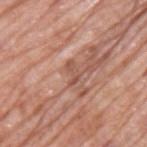Part of a total-body skin-imaging series; this lesion was reviewed on a skin check and was not flagged for biopsy.
A male subject aged 68 to 72.
An algorithmic analysis of the crop reported an area of roughly 3 mm², an outline eccentricity of about 0.85 (0 = round, 1 = elongated), and a shape-asymmetry score of about 0.6 (0 = symmetric). The analysis additionally found a lesion color around L≈53 a*≈23 b*≈28 in CIELAB, about 8 CIELAB-L* units darker than the surrounding skin, and a normalized lesion–skin contrast near 6. And it measured a border-irregularity rating of about 5.5/10 and a color-variation rating of about 0/10. The analysis additionally found a classifier nevus-likeness of about 0/100 and a detector confidence of about 75 out of 100 that the crop contains a lesion.
The lesion is located on the upper back.
Cropped from a whole-body photographic skin survey; the tile spans about 15 mm.